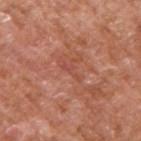biopsy_status: not biopsied; imaged during a skin examination
lighting: white-light
image:
  source: total-body photography crop
  field_of_view_mm: 15
patient:
  sex: male
  age_approx: 65
automated_metrics:
  area_mm2_approx: 2.0
  eccentricity: 0.95
  cielab_L: 49
  cielab_a: 29
  cielab_b: 29
  vs_skin_darker_L: 6.0
  vs_skin_contrast_norm: 4.5
lesion_size:
  long_diameter_mm_approx: 3.0
site: arm